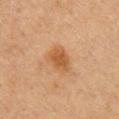follow-up: total-body-photography surveillance lesion; no biopsy.